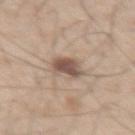Imaged during a routine full-body skin examination; the lesion was not biopsied and no histopathology is available.
Located on the right thigh.
A region of skin cropped from a whole-body photographic capture, roughly 15 mm wide.
The tile uses white-light illumination.
A male subject, about 60 years old.
About 3.5 mm across.